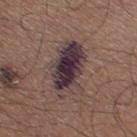Case summary:
* workup: total-body-photography surveillance lesion; no biopsy